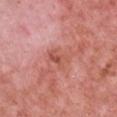Located on the chest. Longest diameter approximately 3 mm. The tile uses white-light illumination. Cropped from a total-body skin-imaging series; the visible field is about 15 mm. Automated tile analysis of the lesion measured a lesion color around L≈54 a*≈28 b*≈30 in CIELAB and about 8 CIELAB-L* units darker than the surrounding skin. And it measured a classifier nevus-likeness of about 0/100 and lesion-presence confidence of about 100/100. A female subject aged approximately 40.